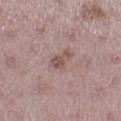biopsy status: catalogued during a skin exam; not biopsied
lighting: white-light illumination
diameter: ≈3 mm
patient: female, approximately 45 years of age
image source: ~15 mm crop, total-body skin-cancer survey
TBP lesion metrics: a normalized lesion–skin contrast near 7; a lesion-detection confidence of about 100/100
anatomic site: the right lower leg